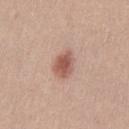No biopsy was performed on this lesion — it was imaged during a full skin examination and was not determined to be concerning. From the left thigh. A 15 mm close-up tile from a total-body photography series done for melanoma screening. The tile uses white-light illumination. A female patient in their 20s. Automated image analysis of the tile measured a lesion area of about 6.5 mm², an eccentricity of roughly 0.75, and two-axis asymmetry of about 0.2. The recorded lesion diameter is about 3.5 mm.